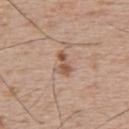This lesion was catalogued during total-body skin photography and was not selected for biopsy.
From the upper back.
Cropped from a total-body skin-imaging series; the visible field is about 15 mm.
Longest diameter approximately 3 mm.
This is a white-light tile.
A male subject aged around 65.